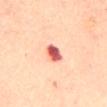  biopsy_status: not biopsied; imaged during a skin examination
  lighting: cross-polarized
  patient:
    sex: female
    age_approx: 45
  site: mid back
  image:
    source: total-body photography crop
    field_of_view_mm: 15